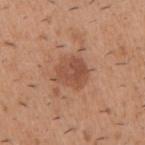Case summary:
- notes · total-body-photography surveillance lesion; no biopsy